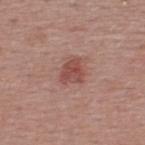<case>
  <automated_metrics>
    <vs_skin_contrast_norm>7.5</vs_skin_contrast_norm>
    <lesion_detection_confidence_0_100>100</lesion_detection_confidence_0_100>
  </automated_metrics>
  <lesion_size>
    <long_diameter_mm_approx>2.5</long_diameter_mm_approx>
  </lesion_size>
  <site>upper back</site>
  <lighting>white-light</lighting>
  <image>
    <source>total-body photography crop</source>
    <field_of_view_mm>15</field_of_view_mm>
  </image>
  <patient>
    <sex>male</sex>
    <age_approx>40</age_approx>
  </patient>
</case>